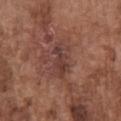This lesion was catalogued during total-body skin photography and was not selected for biopsy.
Measured at roughly 4.5 mm in maximum diameter.
The patient is a male roughly 75 years of age.
Cropped from a total-body skin-imaging series; the visible field is about 15 mm.
This is a white-light tile.
On the chest.
An algorithmic analysis of the crop reported an area of roughly 8.5 mm², an eccentricity of roughly 0.9, and a symmetry-axis asymmetry near 0.25. The software also gave an automated nevus-likeness rating near 0 out of 100.